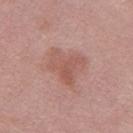Captured during whole-body skin photography for melanoma surveillance; the lesion was not biopsied. Cropped from a total-body skin-imaging series; the visible field is about 15 mm. A female subject, approximately 65 years of age. This is a white-light tile. The recorded lesion diameter is about 4.5 mm. Located on the left thigh. The lesion-visualizer software estimated an eccentricity of roughly 0.55 and a shape-asymmetry score of about 0.45 (0 = symmetric). The software also gave a mean CIELAB color near L≈55 a*≈23 b*≈25, a lesion–skin lightness drop of about 7, and a lesion-to-skin contrast of about 5.5 (normalized; higher = more distinct). And it measured a detector confidence of about 100 out of 100 that the crop contains a lesion.